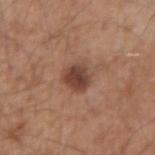follow-up: no biopsy performed (imaged during a skin exam)
subject: male, in their mid- to late 50s
lesion diameter: ≈3.5 mm
lighting: white-light illumination
image source: ~15 mm tile from a whole-body skin photo
location: the arm